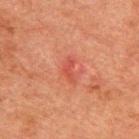Impression: No biopsy was performed on this lesion — it was imaged during a full skin examination and was not determined to be concerning. Background: The lesion is located on the upper back. A male subject approximately 60 years of age. The lesion-visualizer software estimated a lesion color around L≈40 a*≈29 b*≈27 in CIELAB and a lesion-to-skin contrast of about 5.5 (normalized; higher = more distinct). And it measured a border-irregularity rating of about 5/10, internal color variation of about 0 on a 0–10 scale, and peripheral color asymmetry of about 0. This image is a 15 mm lesion crop taken from a total-body photograph. About 3 mm across. This is a cross-polarized tile.